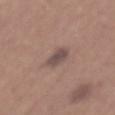workup=total-body-photography surveillance lesion; no biopsy
tile lighting=white-light illumination
diameter=≈3.5 mm
automated lesion analysis=a lesion color around L≈48 a*≈15 b*≈19 in CIELAB, roughly 10 lightness units darker than nearby skin, and a normalized border contrast of about 8.5; an automated nevus-likeness rating near 0 out of 100
subject=male, aged 63–67
image source=~15 mm tile from a whole-body skin photo
site=the back